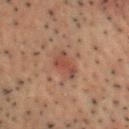Clinical impression:
Imaged during a routine full-body skin examination; the lesion was not biopsied and no histopathology is available.
Context:
A male subject approximately 50 years of age. A 15 mm close-up extracted from a 3D total-body photography capture. Located on the chest. An algorithmic analysis of the crop reported a mean CIELAB color near L≈35 a*≈18 b*≈22, about 7 CIELAB-L* units darker than the surrounding skin, and a lesion-to-skin contrast of about 6.5 (normalized; higher = more distinct). The analysis additionally found an automated nevus-likeness rating near 10 out of 100 and a lesion-detection confidence of about 100/100. Imaged with cross-polarized lighting. The recorded lesion diameter is about 3.5 mm.